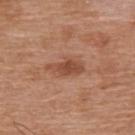The lesion was photographed on a routine skin check and not biopsied; there is no pathology result.
A roughly 15 mm field-of-view crop from a total-body skin photograph.
The lesion-visualizer software estimated a footprint of about 5.5 mm², an outline eccentricity of about 0.9 (0 = round, 1 = elongated), and a symmetry-axis asymmetry near 0.35. It also reported border irregularity of about 4 on a 0–10 scale, internal color variation of about 1.5 on a 0–10 scale, and radial color variation of about 0.5.
The recorded lesion diameter is about 4 mm.
This is a white-light tile.
The lesion is on the upper back.
A male patient, aged 63 to 67.